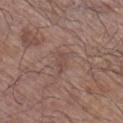Q: Was this lesion biopsied?
A: no biopsy performed (imaged during a skin exam)
Q: What kind of image is this?
A: total-body-photography crop, ~15 mm field of view
Q: What did automated image analysis measure?
A: a footprint of about 3 mm², a shape eccentricity near 0.85, and two-axis asymmetry of about 0.35; a lesion color around L≈47 a*≈18 b*≈22 in CIELAB and a lesion–skin lightness drop of about 6; a nevus-likeness score of about 0/100 and lesion-presence confidence of about 90/100
Q: Illumination type?
A: white-light illumination
Q: How large is the lesion?
A: about 2.5 mm
Q: What are the patient's age and sex?
A: male, approximately 65 years of age
Q: Where on the body is the lesion?
A: the right lower leg